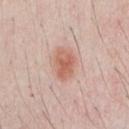Notes:
* notes · imaged on a skin check; not biopsied
* lighting · white-light illumination
* image-analysis metrics · an area of roughly 8 mm² and an eccentricity of roughly 0.75; an average lesion color of about L≈61 a*≈24 b*≈29 (CIELAB), roughly 11 lightness units darker than nearby skin, and a lesion-to-skin contrast of about 7.5 (normalized; higher = more distinct); a border-irregularity index near 2/10, a color-variation rating of about 4.5/10, and peripheral color asymmetry of about 1.5; a classifier nevus-likeness of about 90/100 and a detector confidence of about 100 out of 100 that the crop contains a lesion
* subject · male, aged 23 to 27
* lesion size · ≈4 mm
* imaging modality · 15 mm crop, total-body photography
* anatomic site · the chest